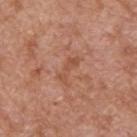This lesion was catalogued during total-body skin photography and was not selected for biopsy.
The lesion's longest dimension is about 3.5 mm.
A male subject about 65 years old.
This image is a 15 mm lesion crop taken from a total-body photograph.
Imaged with white-light lighting.
From the upper back.